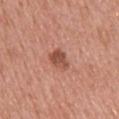The lesion was photographed on a routine skin check and not biopsied; there is no pathology result. The subject is a male roughly 60 years of age. The lesion is located on the back. The lesion-visualizer software estimated an outline eccentricity of about 0.55 (0 = round, 1 = elongated) and a symmetry-axis asymmetry near 0.3. The software also gave a border-irregularity index near 3/10, a color-variation rating of about 3.5/10, and radial color variation of about 1. Longest diameter approximately 2.5 mm. Cropped from a whole-body photographic skin survey; the tile spans about 15 mm. This is a white-light tile.